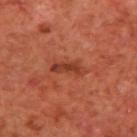Imaged during a routine full-body skin examination; the lesion was not biopsied and no histopathology is available. The lesion's longest dimension is about 4 mm. A male subject aged 68–72. The lesion is located on the upper back. This image is a 15 mm lesion crop taken from a total-body photograph. The total-body-photography lesion software estimated an area of roughly 5 mm², an outline eccentricity of about 0.95 (0 = round, 1 = elongated), and two-axis asymmetry of about 0.4. And it measured a nevus-likeness score of about 5/100 and lesion-presence confidence of about 100/100.